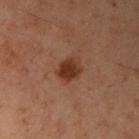Assessment:
This lesion was catalogued during total-body skin photography and was not selected for biopsy.
Background:
Imaged with cross-polarized lighting. This image is a 15 mm lesion crop taken from a total-body photograph. A female subject, aged 53–57. An algorithmic analysis of the crop reported an area of roughly 6 mm², a shape eccentricity near 0.6, and a symmetry-axis asymmetry near 0.15. And it measured border irregularity of about 1.5 on a 0–10 scale, internal color variation of about 3.5 on a 0–10 scale, and peripheral color asymmetry of about 1. From the left arm.